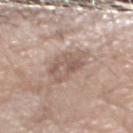Clinical impression:
This lesion was catalogued during total-body skin photography and was not selected for biopsy.
Acquisition and patient details:
The lesion is on the left forearm. A male patient, aged 58 to 62. This image is a 15 mm lesion crop taken from a total-body photograph.